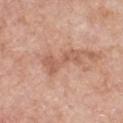<record>
<biopsy_status>not biopsied; imaged during a skin examination</biopsy_status>
<automated_metrics>
  <cielab_L>59</cielab_L>
  <cielab_a>23</cielab_a>
  <cielab_b>30</cielab_b>
  <vs_skin_darker_L>9.0</vs_skin_darker_L>
  <vs_skin_contrast_norm>6.0</vs_skin_contrast_norm>
  <border_irregularity_0_10>6.5</border_irregularity_0_10>
  <color_variation_0_10>2.5</color_variation_0_10>
  <peripheral_color_asymmetry>1.0</peripheral_color_asymmetry>
  <nevus_likeness_0_100>0</nevus_likeness_0_100>
</automated_metrics>
<image>
  <source>total-body photography crop</source>
  <field_of_view_mm>15</field_of_view_mm>
</image>
<site>chest</site>
<patient>
  <sex>male</sex>
  <age_approx>60</age_approx>
</patient>
<lesion_size>
  <long_diameter_mm_approx>5.0</long_diameter_mm_approx>
</lesion_size>
</record>